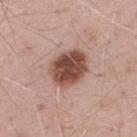The patient is a male approximately 70 years of age.
The lesion is on the upper back.
Cropped from a whole-body photographic skin survey; the tile spans about 15 mm.